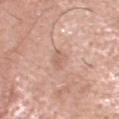Clinical summary: The total-body-photography lesion software estimated an average lesion color of about L≈62 a*≈20 b*≈28 (CIELAB) and roughly 8 lightness units darker than nearby skin. The patient is a male in their mid- to late 60s. A roughly 15 mm field-of-view crop from a total-body skin photograph. On the head or neck.